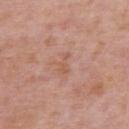Q: Lesion size?
A: ≈2.5 mm
Q: What did automated image analysis measure?
A: a mean CIELAB color near L≈57 a*≈23 b*≈30, about 6 CIELAB-L* units darker than the surrounding skin, and a lesion-to-skin contrast of about 5 (normalized; higher = more distinct)
Q: Lesion location?
A: the upper back
Q: Patient demographics?
A: male, aged 73 to 77
Q: What kind of image is this?
A: ~15 mm crop, total-body skin-cancer survey
Q: How was the tile lit?
A: white-light